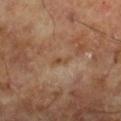{
  "biopsy_status": "not biopsied; imaged during a skin examination",
  "lesion_size": {
    "long_diameter_mm_approx": 2.5
  },
  "lighting": "cross-polarized",
  "automated_metrics": {
    "area_mm2_approx": 2.5,
    "eccentricity": 0.9,
    "cielab_L": 46,
    "cielab_a": 18,
    "cielab_b": 31,
    "vs_skin_darker_L": 6.0,
    "vs_skin_contrast_norm": 5.5
  },
  "patient": {
    "sex": "male",
    "age_approx": 65
  },
  "image": {
    "source": "total-body photography crop",
    "field_of_view_mm": 15
  }
}